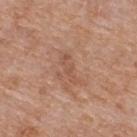No biopsy was performed on this lesion — it was imaged during a full skin examination and was not determined to be concerning. A female patient about 75 years old. From the back. A close-up tile cropped from a whole-body skin photograph, about 15 mm across.